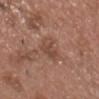* follow-up — imaged on a skin check; not biopsied
* location — the front of the torso
* imaging modality — ~15 mm crop, total-body skin-cancer survey
* illumination — white-light illumination
* lesion diameter — ≈3.5 mm
* subject — male, in their mid-50s